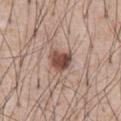| key | value |
|---|---|
| workup | no biopsy performed (imaged during a skin exam) |
| subject | male, approximately 55 years of age |
| location | the chest |
| acquisition | ~15 mm tile from a whole-body skin photo |